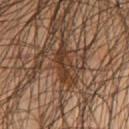follow-up=no biopsy performed (imaged during a skin exam) | lighting=cross-polarized | patient=male, in their mid- to late 50s | lesion size=about 5 mm | location=the chest | imaging modality=~15 mm crop, total-body skin-cancer survey.